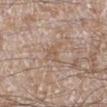  biopsy_status: not biopsied; imaged during a skin examination
  site: left lower leg
  lesion_size:
    long_diameter_mm_approx: 3.0
  lighting: white-light
  image:
    source: total-body photography crop
    field_of_view_mm: 15
  patient:
    sex: male
    age_approx: 60
  automated_metrics:
    shape_asymmetry: 0.35